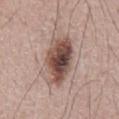Notes:
- workup: no biopsy performed (imaged during a skin exam)
- image-analysis metrics: an area of roughly 19 mm², an outline eccentricity of about 0.8 (0 = round, 1 = elongated), and a shape-asymmetry score of about 0.15 (0 = symmetric); a mean CIELAB color near L≈49 a*≈18 b*≈22 and about 16 CIELAB-L* units darker than the surrounding skin; a within-lesion color-variation index near 9.5/10 and peripheral color asymmetry of about 3; a nevus-likeness score of about 100/100 and a detector confidence of about 100 out of 100 that the crop contains a lesion
- subject: male, approximately 55 years of age
- acquisition: ~15 mm crop, total-body skin-cancer survey